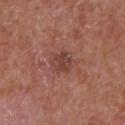patient: male, in their mid- to late 60s | size: about 2.5 mm | TBP lesion metrics: an area of roughly 5 mm², an eccentricity of roughly 0.3, and a shape-asymmetry score of about 0.2 (0 = symmetric); a mean CIELAB color near L≈42 a*≈23 b*≈24, roughly 8 lightness units darker than nearby skin, and a lesion-to-skin contrast of about 6.5 (normalized; higher = more distinct); a lesion-detection confidence of about 100/100 | location: the front of the torso | illumination: white-light | image source: total-body-photography crop, ~15 mm field of view.